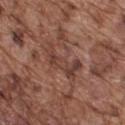Part of a total-body skin-imaging series; this lesion was reviewed on a skin check and was not flagged for biopsy.
A male subject about 75 years old.
A 15 mm close-up tile from a total-body photography series done for melanoma screening.
The lesion is on the upper back.